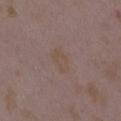Recorded during total-body skin imaging; not selected for excision or biopsy. The lesion's longest dimension is about 3 mm. On the arm. A female subject aged around 35. The tile uses white-light illumination. This image is a 15 mm lesion crop taken from a total-body photograph.